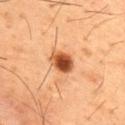{
  "biopsy_status": "not biopsied; imaged during a skin examination",
  "automated_metrics": {
    "cielab_L": 46,
    "cielab_a": 25,
    "cielab_b": 36,
    "vs_skin_darker_L": 17.0,
    "vs_skin_contrast_norm": 12.0
  },
  "patient": {
    "sex": "male",
    "age_approx": 55
  },
  "image": {
    "source": "total-body photography crop",
    "field_of_view_mm": 15
  },
  "lighting": "cross-polarized",
  "lesion_size": {
    "long_diameter_mm_approx": 3.0
  },
  "site": "chest"
}